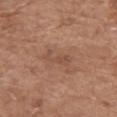workup: imaged on a skin check; not biopsied | size: about 3.5 mm | TBP lesion metrics: a shape eccentricity near 0.95 and two-axis asymmetry of about 0.4; a lesion–skin lightness drop of about 7; a nevus-likeness score of about 0/100 and a detector confidence of about 100 out of 100 that the crop contains a lesion | acquisition: ~15 mm crop, total-body skin-cancer survey | site: the mid back | patient: female, in their mid-70s | lighting: white-light illumination.